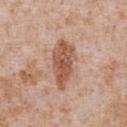Impression:
The lesion was photographed on a routine skin check and not biopsied; there is no pathology result.
Context:
The subject is a male aged 63–67. Automated tile analysis of the lesion measured a footprint of about 13 mm², an outline eccentricity of about 0.9 (0 = round, 1 = elongated), and a shape-asymmetry score of about 0.2 (0 = symmetric). It also reported an automated nevus-likeness rating near 60 out of 100 and lesion-presence confidence of about 100/100. Imaged with white-light lighting. A 15 mm crop from a total-body photograph taken for skin-cancer surveillance. The lesion is on the chest. Longest diameter approximately 6 mm.